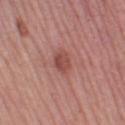Q: Is there a histopathology result?
A: catalogued during a skin exam; not biopsied
Q: Where on the body is the lesion?
A: the left thigh
Q: What is the lesion's diameter?
A: ≈3 mm
Q: What is the imaging modality?
A: ~15 mm crop, total-body skin-cancer survey
Q: Illumination type?
A: white-light
Q: Patient demographics?
A: female, in their mid-40s